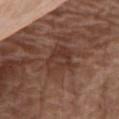Assessment: No biopsy was performed on this lesion — it was imaged during a full skin examination and was not determined to be concerning. Acquisition and patient details: A roughly 15 mm field-of-view crop from a total-body skin photograph. Located on the abdomen. A female patient, aged approximately 75. The lesion-visualizer software estimated a lesion area of about 11 mm², an outline eccentricity of about 0.6 (0 = round, 1 = elongated), and a shape-asymmetry score of about 0.3 (0 = symmetric).